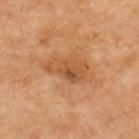Impression:
This lesion was catalogued during total-body skin photography and was not selected for biopsy.
Image and clinical context:
The lesion is on the upper back. A 15 mm close-up extracted from a 3D total-body photography capture. Imaged with cross-polarized lighting. A male subject approximately 70 years of age. The lesion's longest dimension is about 5.5 mm.